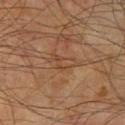{"biopsy_status": "not biopsied; imaged during a skin examination"}